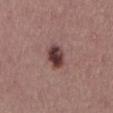Case summary:
* workup: catalogued during a skin exam; not biopsied
* body site: the mid back
* TBP lesion metrics: an area of roughly 6.5 mm² and a shape-asymmetry score of about 0.15 (0 = symmetric); border irregularity of about 2 on a 0–10 scale, a color-variation rating of about 5.5/10, and a peripheral color-asymmetry measure near 2
* illumination: white-light illumination
* lesion diameter: ~3.5 mm (longest diameter)
* subject: male, roughly 40 years of age
* acquisition: total-body-photography crop, ~15 mm field of view